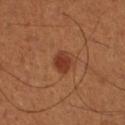Q: Was this lesion biopsied?
A: total-body-photography surveillance lesion; no biopsy
Q: How large is the lesion?
A: about 2.5 mm
Q: What is the imaging modality?
A: 15 mm crop, total-body photography
Q: Lesion location?
A: the leg
Q: Patient demographics?
A: male, aged around 50